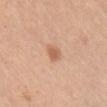Clinical impression: The lesion was tiled from a total-body skin photograph and was not biopsied. Background: A 15 mm close-up extracted from a 3D total-body photography capture. A female patient aged approximately 30. Captured under white-light illumination. An algorithmic analysis of the crop reported an average lesion color of about L≈61 a*≈22 b*≈34 (CIELAB) and roughly 10 lightness units darker than nearby skin. It also reported border irregularity of about 2.5 on a 0–10 scale, a color-variation rating of about 1.5/10, and a peripheral color-asymmetry measure near 0.5. It also reported a nevus-likeness score of about 75/100 and lesion-presence confidence of about 100/100. Located on the chest.